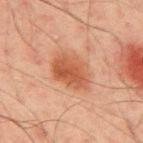follow-up = imaged on a skin check; not biopsied
anatomic site = the mid back
lighting = cross-polarized
acquisition = ~15 mm tile from a whole-body skin photo
patient = male, aged 43–47
TBP lesion metrics = a shape eccentricity near 0.75 and a symmetry-axis asymmetry near 0.15; a border-irregularity index near 2/10, a within-lesion color-variation index near 4/10, and peripheral color asymmetry of about 1.5; a classifier nevus-likeness of about 95/100
lesion size = ~5 mm (longest diameter)